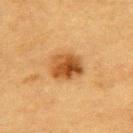Clinical impression: Recorded during total-body skin imaging; not selected for excision or biopsy. Clinical summary: On the back. Measured at roughly 4 mm in maximum diameter. This image is a 15 mm lesion crop taken from a total-body photograph. The tile uses cross-polarized illumination. The subject is a male aged around 85 or older.